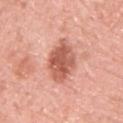A lesion tile, about 15 mm wide, cut from a 3D total-body photograph. On the upper back. The lesion-visualizer software estimated an area of roughly 14 mm², an eccentricity of roughly 0.7, and two-axis asymmetry of about 0.2. It also reported about 13 CIELAB-L* units darker than the surrounding skin and a normalized lesion–skin contrast near 8.5. A male patient, aged 63 to 67.